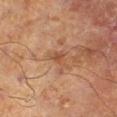{
  "image": {
    "source": "total-body photography crop",
    "field_of_view_mm": 15
  },
  "site": "right lower leg",
  "patient": {
    "sex": "male",
    "age_approx": 70
  }
}